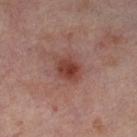Assessment: No biopsy was performed on this lesion — it was imaged during a full skin examination and was not determined to be concerning. Acquisition and patient details: This image is a 15 mm lesion crop taken from a total-body photograph. A female patient aged around 55. On the left thigh.